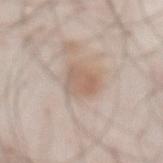No biopsy was performed on this lesion — it was imaged during a full skin examination and was not determined to be concerning. The subject is a male approximately 55 years of age. The tile uses white-light illumination. A 15 mm close-up tile from a total-body photography series done for melanoma screening. The lesion is on the abdomen. An algorithmic analysis of the crop reported a shape eccentricity near 0.35 and a symmetry-axis asymmetry near 0.3. It also reported a lesion–skin lightness drop of about 8 and a normalized border contrast of about 6. It also reported border irregularity of about 3 on a 0–10 scale and internal color variation of about 3 on a 0–10 scale. The lesion's longest dimension is about 3.5 mm.